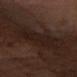Part of a total-body skin-imaging series; this lesion was reviewed on a skin check and was not flagged for biopsy.
The subject is a male aged 58–62.
The lesion's longest dimension is about 4.5 mm.
A 15 mm close-up tile from a total-body photography series done for melanoma screening.
Located on the left forearm.
The lesion-visualizer software estimated a border-irregularity rating of about 4.5/10 and a within-lesion color-variation index near 2.5/10. It also reported a classifier nevus-likeness of about 0/100.